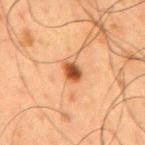<case>
  <biopsy_status>not biopsied; imaged during a skin examination</biopsy_status>
  <lighting>cross-polarized</lighting>
  <patient>
    <sex>male</sex>
    <age_approx>60</age_approx>
  </patient>
  <lesion_size>
    <long_diameter_mm_approx>2.5</long_diameter_mm_approx>
  </lesion_size>
  <site>mid back</site>
  <image>
    <source>total-body photography crop</source>
    <field_of_view_mm>15</field_of_view_mm>
  </image>
  <automated_metrics>
    <area_mm2_approx>4.0</area_mm2_approx>
    <eccentricity>0.75</eccentricity>
    <shape_asymmetry>0.25</shape_asymmetry>
  </automated_metrics>
</case>